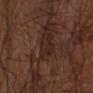{"biopsy_status": "not biopsied; imaged during a skin examination", "image": {"source": "total-body photography crop", "field_of_view_mm": 15}, "site": "left forearm", "lighting": "cross-polarized", "lesion_size": {"long_diameter_mm_approx": 3.5}, "patient": {"sex": "male", "age_approx": 65}}